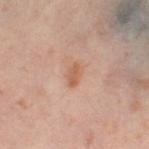• subject · female, approximately 50 years of age
• TBP lesion metrics · a nevus-likeness score of about 20/100 and lesion-presence confidence of about 100/100
• tile lighting · cross-polarized
• image source · 15 mm crop, total-body photography
• location · the right thigh
• lesion diameter · ~2.5 mm (longest diameter)